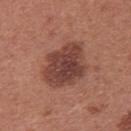Clinical impression: Part of a total-body skin-imaging series; this lesion was reviewed on a skin check and was not flagged for biopsy. Context: Cropped from a whole-body photographic skin survey; the tile spans about 15 mm. This is a white-light tile. Measured at roughly 6 mm in maximum diameter. From the upper back. An algorithmic analysis of the crop reported a lesion area of about 20 mm², a shape eccentricity near 0.65, and a shape-asymmetry score of about 0.2 (0 = symmetric). The analysis additionally found a mean CIELAB color near L≈42 a*≈23 b*≈25 and a normalized border contrast of about 9.5. The patient is a female aged 23–27.